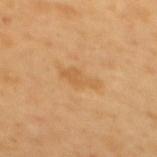Q: Was this lesion biopsied?
A: no biopsy performed (imaged during a skin exam)
Q: What lighting was used for the tile?
A: cross-polarized
Q: Who is the patient?
A: female, in their mid-50s
Q: What did automated image analysis measure?
A: a lesion area of about 5.5 mm², an eccentricity of roughly 0.9, and a symmetry-axis asymmetry near 0.35; a mean CIELAB color near L≈60 a*≈20 b*≈43, a lesion–skin lightness drop of about 7, and a lesion-to-skin contrast of about 5 (normalized; higher = more distinct); a border-irregularity index near 4/10 and radial color variation of about 0.5
Q: How was this image acquired?
A: total-body-photography crop, ~15 mm field of view
Q: What is the anatomic site?
A: the upper back